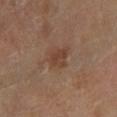<record>
  <biopsy_status>not biopsied; imaged during a skin examination</biopsy_status>
</record>